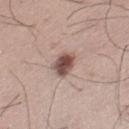Q: Is there a histopathology result?
A: no biopsy performed (imaged during a skin exam)
Q: Illumination type?
A: white-light illumination
Q: What are the patient's age and sex?
A: male, roughly 35 years of age
Q: Automated lesion metrics?
A: a mean CIELAB color near L≈51 a*≈19 b*≈22, about 16 CIELAB-L* units darker than the surrounding skin, and a lesion-to-skin contrast of about 11 (normalized; higher = more distinct); border irregularity of about 1.5 on a 0–10 scale, a within-lesion color-variation index near 6/10, and radial color variation of about 2
Q: What kind of image is this?
A: ~15 mm crop, total-body skin-cancer survey
Q: What is the anatomic site?
A: the left thigh
Q: What is the lesion's diameter?
A: about 3 mm